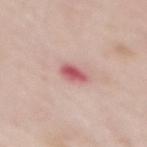follow-up: catalogued during a skin exam; not biopsied | anatomic site: the mid back | subject: female, in their 50s | acquisition: 15 mm crop, total-body photography.